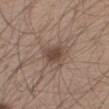Q: Is there a histopathology result?
A: total-body-photography surveillance lesion; no biopsy
Q: Lesion size?
A: about 3.5 mm
Q: What lighting was used for the tile?
A: white-light illumination
Q: Where on the body is the lesion?
A: the leg
Q: Who is the patient?
A: male, approximately 60 years of age
Q: What is the imaging modality?
A: total-body-photography crop, ~15 mm field of view
Q: Automated lesion metrics?
A: a lesion area of about 7 mm² and a shape eccentricity near 0.6; a lesion color around L≈46 a*≈16 b*≈24 in CIELAB, a lesion–skin lightness drop of about 10, and a lesion-to-skin contrast of about 7.5 (normalized; higher = more distinct)